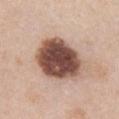Impression: This lesion was catalogued during total-body skin photography and was not selected for biopsy. Acquisition and patient details: The lesion is located on the chest. A 15 mm close-up extracted from a 3D total-body photography capture. A female patient aged approximately 60. Automated image analysis of the tile measured a footprint of about 26 mm², an outline eccentricity of about 0.65 (0 = round, 1 = elongated), and two-axis asymmetry of about 0.15.